The tile uses white-light illumination · on the upper back · the subject is a female roughly 60 years of age · cropped from a whole-body photographic skin survey; the tile spans about 15 mm · the recorded lesion diameter is about 9 mm.
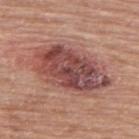Q: What is the histopathologic diagnosis?
A: an invasive melanoma, superficial spreading type; Breslow depth 0.8 mm, mitotic rate 1/mm² — a malignancy